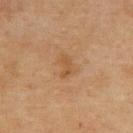workup: total-body-photography surveillance lesion; no biopsy
anatomic site: the upper back
diameter: ~3 mm (longest diameter)
subject: female, aged approximately 55
imaging modality: ~15 mm crop, total-body skin-cancer survey
lighting: cross-polarized illumination